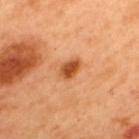Notes:
• follow-up · total-body-photography surveillance lesion; no biopsy
• imaging modality · ~15 mm tile from a whole-body skin photo
• size · ~3 mm (longest diameter)
• automated metrics · a shape eccentricity near 0.75; a mean CIELAB color near L≈52 a*≈30 b*≈44, a lesion–skin lightness drop of about 15, and a normalized lesion–skin contrast near 10; a nevus-likeness score of about 100/100 and a lesion-detection confidence of about 100/100
• tile lighting · cross-polarized illumination
• subject · male, in their 50s
• anatomic site · the upper back